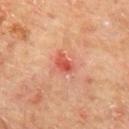• notes: imaged on a skin check; not biopsied
• lighting: cross-polarized illumination
• body site: the mid back
• lesion diameter: about 3 mm
• image: ~15 mm crop, total-body skin-cancer survey
• patient: male, in their mid-60s